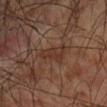Notes:
* image source · 15 mm crop, total-body photography
* diameter · about 4.5 mm
* tile lighting · cross-polarized illumination
* location · the left upper arm
* subject · male, about 75 years old